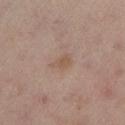Notes:
* biopsy status · no biopsy performed (imaged during a skin exam)
* image source · ~15 mm tile from a whole-body skin photo
* patient · female, about 55 years old
* automated lesion analysis · a footprint of about 3 mm²; an average lesion color of about L≈54 a*≈17 b*≈28 (CIELAB), roughly 6 lightness units darker than nearby skin, and a normalized lesion–skin contrast near 6
* site · the left lower leg